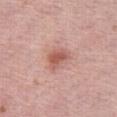Case summary:
• notes · total-body-photography surveillance lesion; no biopsy
• imaging modality · ~15 mm tile from a whole-body skin photo
• lesion diameter · about 3 mm
• location · the right thigh
• patient · female, approximately 65 years of age